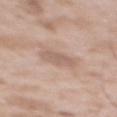Q: Where on the body is the lesion?
A: the back
Q: What did automated image analysis measure?
A: a lesion color around L≈60 a*≈17 b*≈25 in CIELAB, roughly 8 lightness units darker than nearby skin, and a normalized lesion–skin contrast near 5; a border-irregularity rating of about 2/10, internal color variation of about 1.5 on a 0–10 scale, and radial color variation of about 0.5
Q: What is the imaging modality?
A: 15 mm crop, total-body photography
Q: What are the patient's age and sex?
A: male, aged 48 to 52
Q: How large is the lesion?
A: about 3.5 mm